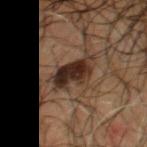Case summary:
• workup: total-body-photography surveillance lesion; no biopsy
• lesion diameter: ≈4.5 mm
• acquisition: 15 mm crop, total-body photography
• patient: male, aged 53 to 57
• site: the chest